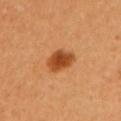Notes:
– notes: imaged on a skin check; not biopsied
– subject: female, aged 48–52
– acquisition: ~15 mm tile from a whole-body skin photo
– illumination: cross-polarized illumination
– diameter: ~4 mm (longest diameter)